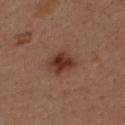Impression:
The lesion was photographed on a routine skin check and not biopsied; there is no pathology result.
Background:
A male patient in their mid-30s. A 15 mm close-up tile from a total-body photography series done for melanoma screening. The lesion's longest dimension is about 4.5 mm. The lesion is located on the back. Imaged with cross-polarized lighting.